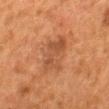Recorded during total-body skin imaging; not selected for excision or biopsy. A female patient in their 50s. Approximately 5.5 mm at its widest. Imaged with cross-polarized lighting. A close-up tile cropped from a whole-body skin photograph, about 15 mm across. Automated image analysis of the tile measured a lesion color around L≈42 a*≈21 b*≈31 in CIELAB and a normalized border contrast of about 5.5. And it measured a border-irregularity index near 3.5/10, a color-variation rating of about 3.5/10, and radial color variation of about 1. It also reported an automated nevus-likeness rating near 0 out of 100 and a lesion-detection confidence of about 100/100. From the mid back.